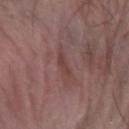notes: total-body-photography surveillance lesion; no biopsy | location: the left forearm | patient: male, approximately 65 years of age | diameter: about 2.5 mm | image: total-body-photography crop, ~15 mm field of view | TBP lesion metrics: a lesion area of about 2.5 mm² and a symmetry-axis asymmetry near 0.35; a mean CIELAB color near L≈41 a*≈20 b*≈21, about 6 CIELAB-L* units darker than the surrounding skin, and a lesion-to-skin contrast of about 6 (normalized; higher = more distinct); peripheral color asymmetry of about 0 | illumination: white-light illumination.